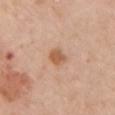Findings:
- image — 15 mm crop, total-body photography
- body site — the left upper arm
- patient — female, aged 63–67
- lesion size — about 2.5 mm
- automated lesion analysis — a border-irregularity index near 1/10, a color-variation rating of about 1.5/10, and a peripheral color-asymmetry measure near 0.5; a lesion-detection confidence of about 100/100